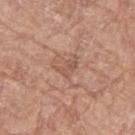Q: Is there a histopathology result?
A: no biopsy performed (imaged during a skin exam)
Q: Patient demographics?
A: female, aged 73–77
Q: What is the lesion's diameter?
A: ≈2.5 mm
Q: What is the imaging modality?
A: ~15 mm crop, total-body skin-cancer survey
Q: Illumination type?
A: white-light illumination
Q: Lesion location?
A: the left thigh